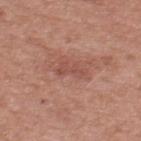workup — total-body-photography surveillance lesion; no biopsy